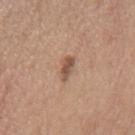No biopsy was performed on this lesion — it was imaged during a full skin examination and was not determined to be concerning.
Measured at roughly 3 mm in maximum diameter.
A lesion tile, about 15 mm wide, cut from a 3D total-body photograph.
Located on the mid back.
Imaged with white-light lighting.
The total-body-photography lesion software estimated an area of roughly 4 mm², a shape eccentricity near 0.9, and a symmetry-axis asymmetry near 0.3. The analysis additionally found a lesion color around L≈53 a*≈19 b*≈28 in CIELAB, about 11 CIELAB-L* units darker than the surrounding skin, and a normalized border contrast of about 8. It also reported border irregularity of about 3.5 on a 0–10 scale and a within-lesion color-variation index near 3.5/10. The software also gave a classifier nevus-likeness of about 85/100 and lesion-presence confidence of about 100/100.
The patient is a female aged 63–67.